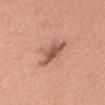{"biopsy_status": "not biopsied; imaged during a skin examination", "lesion_size": {"long_diameter_mm_approx": 4.5}, "patient": {"sex": "female", "age_approx": 35}, "lighting": "white-light", "image": {"source": "total-body photography crop", "field_of_view_mm": 15}, "site": "head or neck"}